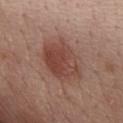| field | value |
|---|---|
| subject | female, about 50 years old |
| body site | the chest |
| acquisition | 15 mm crop, total-body photography |
| lesion size | about 6.5 mm |